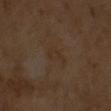Recorded during total-body skin imaging; not selected for excision or biopsy. A 15 mm close-up extracted from a 3D total-body photography capture. A male patient about 65 years old. Captured under cross-polarized illumination. About 3 mm across.